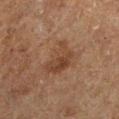Notes:
- follow-up · catalogued during a skin exam; not biopsied
- body site · the left lower leg
- subject · male, about 65 years old
- diameter · ~4.5 mm (longest diameter)
- image-analysis metrics · an area of roughly 12 mm² and an outline eccentricity of about 0.5 (0 = round, 1 = elongated); an average lesion color of about L≈34 a*≈16 b*≈25 (CIELAB) and roughly 6 lightness units darker than nearby skin; a color-variation rating of about 4/10 and peripheral color asymmetry of about 1.5; a classifier nevus-likeness of about 5/100 and a detector confidence of about 100 out of 100 that the crop contains a lesion
- imaging modality · ~15 mm crop, total-body skin-cancer survey